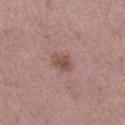Impression: This lesion was catalogued during total-body skin photography and was not selected for biopsy. Acquisition and patient details: This image is a 15 mm lesion crop taken from a total-body photograph. Approximately 3 mm at its widest. The lesion is located on the right lower leg. Imaged with white-light lighting. The subject is a female roughly 40 years of age.